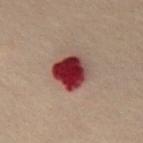{
  "biopsy_status": "not biopsied; imaged during a skin examination",
  "image": {
    "source": "total-body photography crop",
    "field_of_view_mm": 15
  },
  "lesion_size": {
    "long_diameter_mm_approx": 4.0
  },
  "automated_metrics": {
    "area_mm2_approx": 12.0,
    "shape_asymmetry": 0.2,
    "cielab_L": 29,
    "cielab_a": 28,
    "cielab_b": 19,
    "vs_skin_darker_L": 20.0,
    "vs_skin_contrast_norm": 17.0,
    "border_irregularity_0_10": 2.0,
    "color_variation_0_10": 5.5,
    "peripheral_color_asymmetry": 1.5
  },
  "patient": {
    "sex": "male",
    "age_approx": 50
  },
  "site": "abdomen",
  "lighting": "cross-polarized"
}